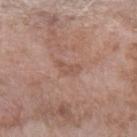Captured during whole-body skin photography for melanoma surveillance; the lesion was not biopsied. This is a white-light tile. The lesion's longest dimension is about 3 mm. A 15 mm close-up tile from a total-body photography series done for melanoma screening. On the arm. A female patient, aged approximately 75.